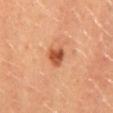Findings:
– notes · no biopsy performed (imaged during a skin exam)